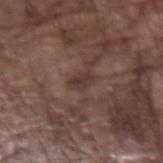| field | value |
|---|---|
| notes | imaged on a skin check; not biopsied |
| size | ≈2.5 mm |
| acquisition | total-body-photography crop, ~15 mm field of view |
| patient | male, in their mid-70s |
| tile lighting | white-light |
| location | the left forearm |